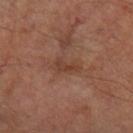workup: total-body-photography surveillance lesion; no biopsy | image: 15 mm crop, total-body photography | tile lighting: cross-polarized illumination | diameter: about 3 mm | subject: male, approximately 70 years of age | automated metrics: an automated nevus-likeness rating near 0 out of 100 | body site: the right thigh.